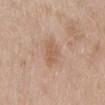biopsy_status: not biopsied; imaged during a skin examination
image:
  source: total-body photography crop
  field_of_view_mm: 15
lesion_size:
  long_diameter_mm_approx: 4.5
patient:
  sex: female
  age_approx: 60
lighting: white-light
site: back
automated_metrics:
  cielab_L: 59
  cielab_a: 18
  cielab_b: 30
  vs_skin_darker_L: 7.0
  vs_skin_contrast_norm: 5.5